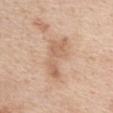The lesion was tiled from a total-body skin photograph and was not biopsied. Imaged with white-light lighting. From the front of the torso. The lesion-visualizer software estimated a lesion area of about 9.5 mm² and a shape eccentricity near 0.9. The software also gave a nevus-likeness score of about 0/100 and a lesion-detection confidence of about 100/100. A female patient, approximately 40 years of age. A 15 mm close-up extracted from a 3D total-body photography capture. About 5.5 mm across.